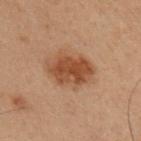Background: This image is a 15 mm lesion crop taken from a total-body photograph. The subject is a male aged 53–57. The lesion is located on the right upper arm. Approximately 4.5 mm at its widest.